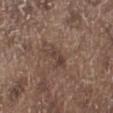workup: imaged on a skin check; not biopsied | subject: male, approximately 70 years of age | site: the left lower leg | tile lighting: white-light illumination | image: ~15 mm crop, total-body skin-cancer survey.